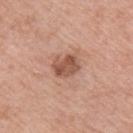The lesion was tiled from a total-body skin photograph and was not biopsied.
On the right upper arm.
The patient is a male aged 58–62.
A 15 mm crop from a total-body photograph taken for skin-cancer surveillance.